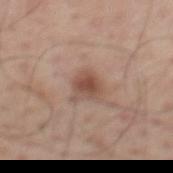This lesion was catalogued during total-body skin photography and was not selected for biopsy. A male subject aged 58–62. A roughly 15 mm field-of-view crop from a total-body skin photograph. From the mid back.